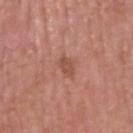{
  "biopsy_status": "not biopsied; imaged during a skin examination",
  "image": {
    "source": "total-body photography crop",
    "field_of_view_mm": 15
  },
  "lesion_size": {
    "long_diameter_mm_approx": 2.5
  },
  "patient": {
    "sex": "male",
    "age_approx": 55
  },
  "site": "head or neck"
}